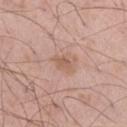notes = imaged on a skin check; not biopsied | anatomic site = the right thigh | subject = male, aged around 55 | image source = total-body-photography crop, ~15 mm field of view.